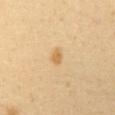Impression: Part of a total-body skin-imaging series; this lesion was reviewed on a skin check and was not flagged for biopsy. Image and clinical context: Cropped from a whole-body photographic skin survey; the tile spans about 15 mm. A female patient roughly 50 years of age. The lesion is located on the abdomen.